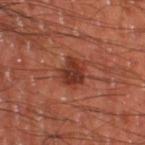Q: Is there a histopathology result?
A: total-body-photography surveillance lesion; no biopsy
Q: Where on the body is the lesion?
A: the left thigh
Q: What did automated image analysis measure?
A: border irregularity of about 3 on a 0–10 scale, internal color variation of about 3.5 on a 0–10 scale, and a peripheral color-asymmetry measure near 1; a nevus-likeness score of about 80/100 and a lesion-detection confidence of about 100/100
Q: How was this image acquired?
A: 15 mm crop, total-body photography
Q: What are the patient's age and sex?
A: male, aged approximately 60
Q: Illumination type?
A: cross-polarized illumination
Q: How large is the lesion?
A: ~3.5 mm (longest diameter)